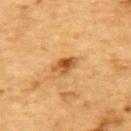workup: no biopsy performed (imaged during a skin exam) | patient: male, in their mid-80s or older | illumination: cross-polarized illumination | TBP lesion metrics: an eccentricity of roughly 0.8 and two-axis asymmetry of about 0.25; a mean CIELAB color near L≈48 a*≈22 b*≈40, roughly 11 lightness units darker than nearby skin, and a normalized border contrast of about 8.5; a nevus-likeness score of about 80/100 and lesion-presence confidence of about 100/100 | image: 15 mm crop, total-body photography | location: the back | lesion size: ≈3 mm.